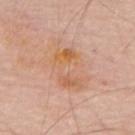workup=total-body-photography surveillance lesion; no biopsy
illumination=white-light
image=~15 mm crop, total-body skin-cancer survey
subject=male, in their 70s
diameter=~6.5 mm (longest diameter)
body site=the upper back